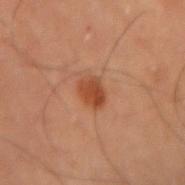Clinical summary: A male patient in their mid- to late 50s. A 15 mm close-up tile from a total-body photography series done for melanoma screening. The lesion is on the arm.